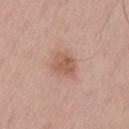biopsy status = catalogued during a skin exam; not biopsied
subject = male, in their mid-50s
image = 15 mm crop, total-body photography
lesion size = ≈3 mm
illumination = white-light
site = the lower back